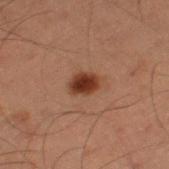biopsy status: catalogued during a skin exam; not biopsied
tile lighting: cross-polarized illumination
body site: the left thigh
patient: male, aged approximately 60
size: about 3 mm
image source: ~15 mm crop, total-body skin-cancer survey